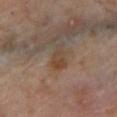biopsy_status: not biopsied; imaged during a skin examination
automated_metrics:
  eccentricity: 0.8
  vs_skin_darker_L: 6.0
  vs_skin_contrast_norm: 7.0
  color_variation_0_10: 4.0
  peripheral_color_asymmetry: 1.5
  nevus_likeness_0_100: 0
  lesion_detection_confidence_0_100: 100
site: left lower leg
image:
  source: total-body photography crop
  field_of_view_mm: 15
lighting: cross-polarized
patient:
  sex: male
  age_approx: 70